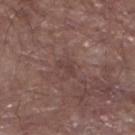notes: no biopsy performed (imaged during a skin exam); imaging modality: total-body-photography crop, ~15 mm field of view; size: about 2.5 mm; patient: male, about 80 years old; lighting: white-light illumination; anatomic site: the left lower leg.